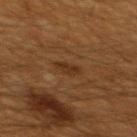workup: no biopsy performed (imaged during a skin exam); imaging modality: ~15 mm crop, total-body skin-cancer survey; illumination: cross-polarized; lesion diameter: ~2.5 mm (longest diameter); subject: male, aged around 60; automated metrics: a lesion color around L≈29 a*≈19 b*≈30 in CIELAB and about 6 CIELAB-L* units darker than the surrounding skin; anatomic site: the mid back.